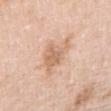Recorded during total-body skin imaging; not selected for excision or biopsy. A 15 mm close-up extracted from a 3D total-body photography capture. This is a white-light tile. On the arm. Automated tile analysis of the lesion measured a color-variation rating of about 3.5/10. The patient is a female aged 43 to 47.